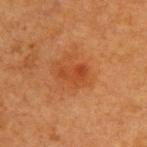Assessment: The lesion was tiled from a total-body skin photograph and was not biopsied. Clinical summary: A female subject, in their 40s. The lesion is on the upper back. A lesion tile, about 15 mm wide, cut from a 3D total-body photograph. Approximately 3 mm at its widest. Automated tile analysis of the lesion measured a border-irregularity index near 3.5/10, a within-lesion color-variation index near 3/10, and peripheral color asymmetry of about 1. The tile uses cross-polarized illumination.